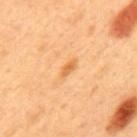Recorded during total-body skin imaging; not selected for excision or biopsy. Cropped from a whole-body photographic skin survey; the tile spans about 15 mm. Imaged with cross-polarized lighting. The patient is a male roughly 50 years of age. Measured at roughly 2.5 mm in maximum diameter. The lesion is on the back.